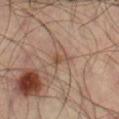Case summary:
– size · about 2.5 mm
– automated lesion analysis · an eccentricity of roughly 0.75 and a symmetry-axis asymmetry near 0.55; a color-variation rating of about 1/10 and peripheral color asymmetry of about 0.5; a nevus-likeness score of about 0/100
– lighting · cross-polarized
– image · 15 mm crop, total-body photography
– anatomic site · the right thigh